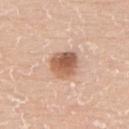Part of a total-body skin-imaging series; this lesion was reviewed on a skin check and was not flagged for biopsy. The lesion's longest dimension is about 4 mm. Captured under white-light illumination. A male patient, approximately 60 years of age. Located on the upper back. A 15 mm close-up tile from a total-body photography series done for melanoma screening.